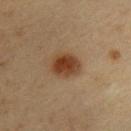This lesion was catalogued during total-body skin photography and was not selected for biopsy. Cropped from a total-body skin-imaging series; the visible field is about 15 mm. On the upper back. Measured at roughly 4 mm in maximum diameter. Imaged with cross-polarized lighting. The subject is a male about 40 years old. Automated tile analysis of the lesion measured border irregularity of about 1.5 on a 0–10 scale, internal color variation of about 4.5 on a 0–10 scale, and peripheral color asymmetry of about 1.